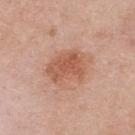Q: Was this lesion biopsied?
A: no biopsy performed (imaged during a skin exam)
Q: How large is the lesion?
A: about 5.5 mm
Q: What are the patient's age and sex?
A: female, aged approximately 40
Q: Lesion location?
A: the upper back
Q: Automated lesion metrics?
A: about 10 CIELAB-L* units darker than the surrounding skin and a normalized lesion–skin contrast near 7; border irregularity of about 3.5 on a 0–10 scale, a color-variation rating of about 3/10, and peripheral color asymmetry of about 1
Q: What is the imaging modality?
A: ~15 mm tile from a whole-body skin photo